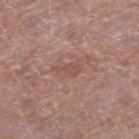{"biopsy_status": "not biopsied; imaged during a skin examination", "image": {"source": "total-body photography crop", "field_of_view_mm": 15}, "patient": {"sex": "male", "age_approx": 65}, "site": "left thigh", "lighting": "white-light"}